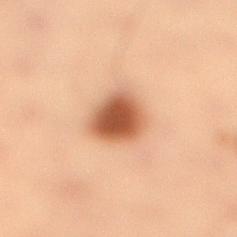A male patient, about 55 years old. Located on the right lower leg. Longest diameter approximately 4 mm. This image is a 15 mm lesion crop taken from a total-body photograph. Automated image analysis of the tile measured a lesion color around L≈45 a*≈22 b*≈31 in CIELAB, a lesion–skin lightness drop of about 16, and a lesion-to-skin contrast of about 11.5 (normalized; higher = more distinct). It also reported a nevus-likeness score of about 100/100 and lesion-presence confidence of about 100/100. Captured under cross-polarized illumination.